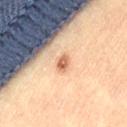{"biopsy_status": "not biopsied; imaged during a skin examination", "site": "left thigh", "lighting": "cross-polarized", "image": {"source": "total-body photography crop", "field_of_view_mm": 15}, "patient": {"sex": "female", "age_approx": 45}, "lesion_size": {"long_diameter_mm_approx": 2.0}}